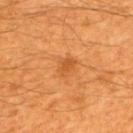Case summary:
* patient: male, aged around 60
* acquisition: total-body-photography crop, ~15 mm field of view
* body site: the mid back
* automated lesion analysis: roughly 8 lightness units darker than nearby skin and a normalized lesion–skin contrast near 5.5; a border-irregularity rating of about 3/10, a color-variation rating of about 2/10, and peripheral color asymmetry of about 0.5; a classifier nevus-likeness of about 30/100 and lesion-presence confidence of about 100/100
* lesion diameter: ≈2.5 mm